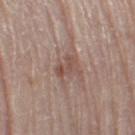Recorded during total-body skin imaging; not selected for excision or biopsy. Located on the left thigh. Approximately 3 mm at its widest. The patient is a male aged approximately 80. A roughly 15 mm field-of-view crop from a total-body skin photograph.